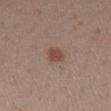– biopsy status — no biopsy performed (imaged during a skin exam)
– tile lighting — white-light
– body site — the leg
– image — ~15 mm crop, total-body skin-cancer survey
– patient — female, aged 28–32
– diameter — about 3 mm